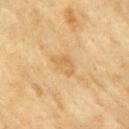Impression:
No biopsy was performed on this lesion — it was imaged during a full skin examination and was not determined to be concerning.
Context:
A region of skin cropped from a whole-body photographic capture, roughly 15 mm wide. The subject is a female aged 58 to 62. The lesion is on the right upper arm. Measured at roughly 3 mm in maximum diameter. This is a cross-polarized tile.